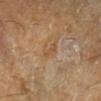biopsy status = imaged on a skin check; not biopsied
location = the left lower leg
subject = male, roughly 60 years of age
image = ~15 mm tile from a whole-body skin photo